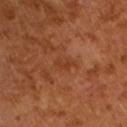  biopsy_status: not biopsied; imaged during a skin examination
  patient:
    sex: male
    age_approx: 65
  lighting: cross-polarized
  image:
    source: total-body photography crop
    field_of_view_mm: 15
  lesion_size:
    long_diameter_mm_approx: 2.5